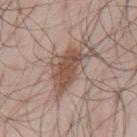From the mid back.
The recorded lesion diameter is about 6.5 mm.
The patient is a male in their mid-40s.
A lesion tile, about 15 mm wide, cut from a 3D total-body photograph.